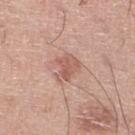Assessment: Recorded during total-body skin imaging; not selected for excision or biopsy. Clinical summary: Located on the right lower leg. The subject is a male in their 20s. A close-up tile cropped from a whole-body skin photograph, about 15 mm across.